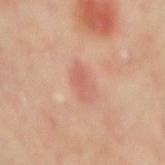A 15 mm crop from a total-body photograph taken for skin-cancer surveillance. Located on the mid back. An algorithmic analysis of the crop reported an outline eccentricity of about 0.85 (0 = round, 1 = elongated) and a symmetry-axis asymmetry near 0.3. The analysis additionally found a lesion color around L≈59 a*≈25 b*≈28 in CIELAB and a normalized lesion–skin contrast near 5. And it measured a border-irregularity rating of about 3/10 and a within-lesion color-variation index near 2.5/10. A male patient, roughly 65 years of age. About 3.5 mm across.